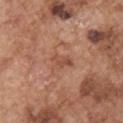Recorded during total-body skin imaging; not selected for excision or biopsy. The tile uses white-light illumination. Measured at roughly 3 mm in maximum diameter. Located on the arm. A 15 mm close-up extracted from a 3D total-body photography capture. The lesion-visualizer software estimated a footprint of about 4 mm² and an outline eccentricity of about 0.75 (0 = round, 1 = elongated). The analysis additionally found a mean CIELAB color near L≈51 a*≈24 b*≈31 and a normalized border contrast of about 5.5. The analysis additionally found a nevus-likeness score of about 0/100 and lesion-presence confidence of about 100/100. The subject is a male aged approximately 75.